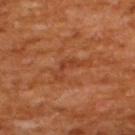{"biopsy_status": "not biopsied; imaged during a skin examination", "lighting": "cross-polarized", "patient": {"sex": "male", "age_approx": 65}, "site": "upper back", "image": {"source": "total-body photography crop", "field_of_view_mm": 15}}